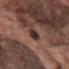Recorded during total-body skin imaging; not selected for excision or biopsy.
A close-up tile cropped from a whole-body skin photograph, about 15 mm across.
The lesion is located on the upper back.
Imaged with white-light lighting.
A male subject, aged approximately 70.